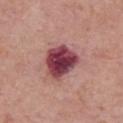Recorded during total-body skin imaging; not selected for excision or biopsy.
On the upper back.
The subject is a female roughly 65 years of age.
This image is a 15 mm lesion crop taken from a total-body photograph.